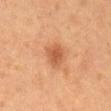workup — catalogued during a skin exam; not biopsied | acquisition — ~15 mm tile from a whole-body skin photo | subject — male, aged 53–57 | anatomic site — the mid back | illumination — cross-polarized | lesion diameter — ~3 mm (longest diameter).